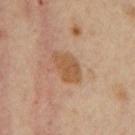Impression: The lesion was photographed on a routine skin check and not biopsied; there is no pathology result. Image and clinical context: The lesion's longest dimension is about 4.5 mm. The subject is a female aged 43 to 47. Located on the mid back. A roughly 15 mm field-of-view crop from a total-body skin photograph.